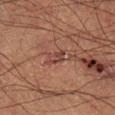Part of a total-body skin-imaging series; this lesion was reviewed on a skin check and was not flagged for biopsy.
Measured at roughly 2.5 mm in maximum diameter.
Imaged with cross-polarized lighting.
A male subject aged approximately 55.
The lesion is on the right lower leg.
A region of skin cropped from a whole-body photographic capture, roughly 15 mm wide.